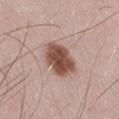lighting=white-light
patient=male, aged approximately 50
image source=~15 mm crop, total-body skin-cancer survey
site=the leg
lesion size=~4.5 mm (longest diameter)